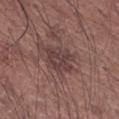notes: catalogued during a skin exam; not biopsied | tile lighting: white-light illumination | automated lesion analysis: a lesion area of about 10 mm² and a shape eccentricity near 0.6; a lesion color around L≈40 a*≈18 b*≈18 in CIELAB, roughly 8 lightness units darker than nearby skin, and a lesion-to-skin contrast of about 7.5 (normalized; higher = more distinct); a border-irregularity index near 3.5/10 and a within-lesion color-variation index near 3/10; a nevus-likeness score of about 0/100 and lesion-presence confidence of about 95/100 | imaging modality: total-body-photography crop, ~15 mm field of view | site: the right upper arm | patient: male, about 65 years old.